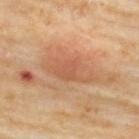No biopsy was performed on this lesion — it was imaged during a full skin examination and was not determined to be concerning. Longest diameter approximately 6.5 mm. The lesion-visualizer software estimated an average lesion color of about L≈57 a*≈23 b*≈35 (CIELAB), a lesion–skin lightness drop of about 9, and a normalized lesion–skin contrast near 6. The software also gave a border-irregularity rating of about 3/10, a color-variation rating of about 3.5/10, and radial color variation of about 1. This image is a 15 mm lesion crop taken from a total-body photograph. A male subject aged 68–72. The tile uses cross-polarized illumination. The lesion is located on the upper back.